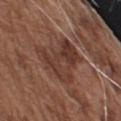This lesion was catalogued during total-body skin photography and was not selected for biopsy.
The lesion is on the left upper arm.
The lesion-visualizer software estimated a border-irregularity rating of about 7/10, a color-variation rating of about 5.5/10, and radial color variation of about 2.
This is a white-light tile.
A male subject aged approximately 75.
A 15 mm crop from a total-body photograph taken for skin-cancer surveillance.